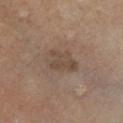| field | value |
|---|---|
| biopsy status | total-body-photography surveillance lesion; no biopsy |
| patient | male, approximately 65 years of age |
| lesion size | ~4 mm (longest diameter) |
| automated lesion analysis | an average lesion color of about L≈43 a*≈13 b*≈24 (CIELAB), about 7 CIELAB-L* units darker than the surrounding skin, and a normalized border contrast of about 6; border irregularity of about 3 on a 0–10 scale, a within-lesion color-variation index near 3/10, and radial color variation of about 1 |
| imaging modality | total-body-photography crop, ~15 mm field of view |
| site | the left lower leg |